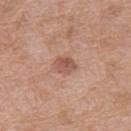No biopsy was performed on this lesion — it was imaged during a full skin examination and was not determined to be concerning. Measured at roughly 2.5 mm in maximum diameter. The tile uses white-light illumination. This image is a 15 mm lesion crop taken from a total-body photograph. Automated tile analysis of the lesion measured a border-irregularity rating of about 2/10 and radial color variation of about 1. It also reported a nevus-likeness score of about 60/100 and a lesion-detection confidence of about 100/100. A female patient, in their 40s. The lesion is on the back.